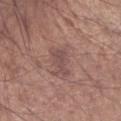  biopsy_status: not biopsied; imaged during a skin examination
  lighting: white-light
  automated_metrics:
    border_irregularity_0_10: 5.5
    color_variation_0_10: 2.0
    nevus_likeness_0_100: 0
    lesion_detection_confidence_0_100: 100
  patient:
    sex: male
    age_approx: 55
  image:
    source: total-body photography crop
    field_of_view_mm: 15
  lesion_size:
    long_diameter_mm_approx: 3.5
  site: right forearm